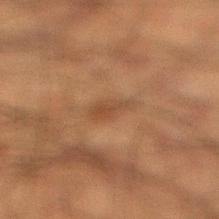The lesion was photographed on a routine skin check and not biopsied; there is no pathology result.
The lesion is on the right lower leg.
A male patient, aged around 50.
A 15 mm crop from a total-body photograph taken for skin-cancer surveillance.